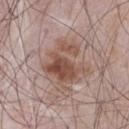The lesion was tiled from a total-body skin photograph and was not biopsied. About 5 mm across. A male patient in their mid- to late 60s. A 15 mm close-up extracted from a 3D total-body photography capture. On the chest. An algorithmic analysis of the crop reported two-axis asymmetry of about 0.5. And it measured an average lesion color of about L≈50 a*≈19 b*≈25 (CIELAB) and about 10 CIELAB-L* units darker than the surrounding skin.